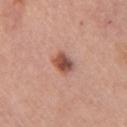The lesion was photographed on a routine skin check and not biopsied; there is no pathology result.
Approximately 3 mm at its widest.
The tile uses white-light illumination.
Automated image analysis of the tile measured a border-irregularity rating of about 2/10 and a within-lesion color-variation index near 6/10.
A female subject aged approximately 60.
A 15 mm close-up extracted from a 3D total-body photography capture.
Located on the right upper arm.